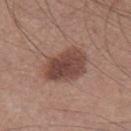Part of a total-body skin-imaging series; this lesion was reviewed on a skin check and was not flagged for biopsy. Automated image analysis of the tile measured a lesion color around L≈45 a*≈20 b*≈24 in CIELAB, about 12 CIELAB-L* units darker than the surrounding skin, and a normalized border contrast of about 9. A lesion tile, about 15 mm wide, cut from a 3D total-body photograph. A male subject aged 43–47. Located on the right thigh. About 5.5 mm across.